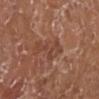Notes:
- follow-up · total-body-photography surveillance lesion; no biopsy
- acquisition · 15 mm crop, total-body photography
- site · the left lower leg
- patient · female, approximately 75 years of age
- automated lesion analysis · a color-variation rating of about 3/10 and a peripheral color-asymmetry measure near 1
- lighting · white-light
- diameter · ≈5 mm